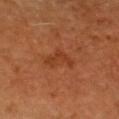No biopsy was performed on this lesion — it was imaged during a full skin examination and was not determined to be concerning. A male subject about 60 years old. The tile uses cross-polarized illumination. Located on the head or neck. About 3.5 mm across. This image is a 15 mm lesion crop taken from a total-body photograph.